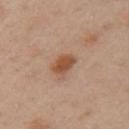From the arm.
A region of skin cropped from a whole-body photographic capture, roughly 15 mm wide.
About 3 mm across.
A female patient in their 40s.
Captured under cross-polarized illumination.